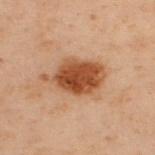Q: What is the imaging modality?
A: total-body-photography crop, ~15 mm field of view
Q: Lesion size?
A: ≈6.5 mm
Q: What is the anatomic site?
A: the back
Q: What are the patient's age and sex?
A: male, about 50 years old